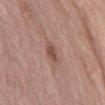| feature | finding |
|---|---|
| workup | catalogued during a skin exam; not biopsied |
| image source | total-body-photography crop, ~15 mm field of view |
| location | the front of the torso |
| subject | male, aged 68–72 |